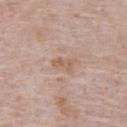Recorded during total-body skin imaging; not selected for excision or biopsy.
A roughly 15 mm field-of-view crop from a total-body skin photograph.
Longest diameter approximately 2.5 mm.
Imaged with white-light lighting.
On the front of the torso.
The subject is a male aged 68 to 72.